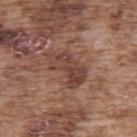Acquisition and patient details: Automated tile analysis of the lesion measured a mean CIELAB color near L≈42 a*≈20 b*≈25. The analysis additionally found border irregularity of about 4 on a 0–10 scale, a within-lesion color-variation index near 4.5/10, and peripheral color asymmetry of about 1.5. The analysis additionally found a nevus-likeness score of about 0/100 and a detector confidence of about 100 out of 100 that the crop contains a lesion. Located on the upper back. A region of skin cropped from a whole-body photographic capture, roughly 15 mm wide. About 5 mm across. The subject is a male in their mid-70s.